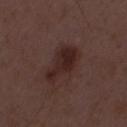follow-up=no biopsy performed (imaged during a skin exam)
tile lighting=white-light
patient=male, in their 50s
diameter=about 5 mm
image source=15 mm crop, total-body photography